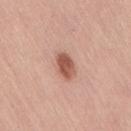The lesion was tiled from a total-body skin photograph and was not biopsied.
An algorithmic analysis of the crop reported a nevus-likeness score of about 100/100 and a lesion-detection confidence of about 100/100.
The lesion's longest dimension is about 3.5 mm.
This is a white-light tile.
On the leg.
This image is a 15 mm lesion crop taken from a total-body photograph.
The patient is a female in their 50s.